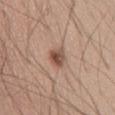Notes:
• notes — total-body-photography surveillance lesion; no biopsy
• lesion diameter — ≈2.5 mm
• anatomic site — the front of the torso
• patient — male, aged approximately 45
• imaging modality — 15 mm crop, total-body photography